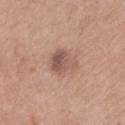The lesion was tiled from a total-body skin photograph and was not biopsied. A 15 mm crop from a total-body photograph taken for skin-cancer surveillance. On the chest. The patient is a female aged around 65.